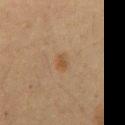This lesion was catalogued during total-body skin photography and was not selected for biopsy.